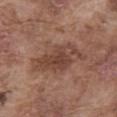  biopsy_status: not biopsied; imaged during a skin examination
  lesion_size:
    long_diameter_mm_approx: 6.5
  lighting: white-light
  automated_metrics:
    eccentricity: 0.9
    cielab_L: 43
    cielab_a: 20
    cielab_b: 26
    border_irregularity_0_10: 5.0
    color_variation_0_10: 4.0
    nevus_likeness_0_100: 20
    lesion_detection_confidence_0_100: 100
  patient:
    sex: male
    age_approx: 75
  site: abdomen
  image:
    source: total-body photography crop
    field_of_view_mm: 15